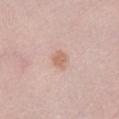Impression:
This lesion was catalogued during total-body skin photography and was not selected for biopsy.